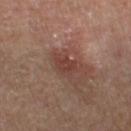  site: leg
  patient:
    sex: male
    age_approx: 85+
  image:
    source: total-body photography crop
    field_of_view_mm: 15
  automated_metrics:
    eccentricity: 0.75
    shape_asymmetry: 0.25
    cielab_L: 38
    cielab_a: 21
    cielab_b: 23
    vs_skin_darker_L: 7.0
    vs_skin_contrast_norm: 6.5
    border_irregularity_0_10: 3.0
    color_variation_0_10: 2.5
    peripheral_color_asymmetry: 1.0
    nevus_likeness_0_100: 10
    lesion_detection_confidence_0_100: 100